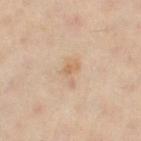This lesion was catalogued during total-body skin photography and was not selected for biopsy. Cropped from a whole-body photographic skin survey; the tile spans about 15 mm. A female subject, approximately 45 years of age. On the left thigh. About 3 mm across. Captured under cross-polarized illumination. The total-body-photography lesion software estimated a symmetry-axis asymmetry near 0.45. The software also gave roughly 7 lightness units darker than nearby skin and a lesion-to-skin contrast of about 5.5 (normalized; higher = more distinct).